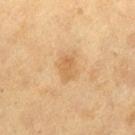Imaged during a routine full-body skin examination; the lesion was not biopsied and no histopathology is available.
This image is a 15 mm lesion crop taken from a total-body photograph.
The lesion is on the leg.
The patient is a female aged 38 to 42.
The lesion-visualizer software estimated a classifier nevus-likeness of about 0/100 and a detector confidence of about 100 out of 100 that the crop contains a lesion.
Captured under cross-polarized illumination.
About 3 mm across.